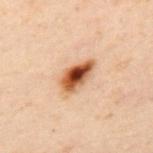Impression:
Imaged during a routine full-body skin examination; the lesion was not biopsied and no histopathology is available.
Acquisition and patient details:
Located on the upper back. A lesion tile, about 15 mm wide, cut from a 3D total-body photograph. Captured under cross-polarized illumination. A male patient, in their 50s.